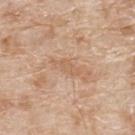Clinical impression:
No biopsy was performed on this lesion — it was imaged during a full skin examination and was not determined to be concerning.
Clinical summary:
The recorded lesion diameter is about 3.5 mm. A male patient aged approximately 80. On the back. A 15 mm close-up extracted from a 3D total-body photography capture.